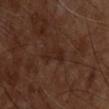{"biopsy_status": "not biopsied; imaged during a skin examination", "lighting": "cross-polarized", "image": {"source": "total-body photography crop", "field_of_view_mm": 15}, "patient": {"sex": "male", "age_approx": 55}, "lesion_size": {"long_diameter_mm_approx": 4.0}, "site": "upper back", "automated_metrics": {"area_mm2_approx": 5.0, "eccentricity": 0.9, "border_irregularity_0_10": 4.5, "color_variation_0_10": 1.0, "peripheral_color_asymmetry": 0.5, "nevus_likeness_0_100": 0, "lesion_detection_confidence_0_100": 100}}